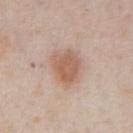This lesion was catalogued during total-body skin photography and was not selected for biopsy.
A roughly 15 mm field-of-view crop from a total-body skin photograph.
The lesion is located on the chest.
A male subject, in their 60s.
Captured under white-light illumination.
Approximately 4.5 mm at its widest.